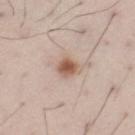A 15 mm crop from a total-body photograph taken for skin-cancer surveillance. The recorded lesion diameter is about 2.5 mm. Imaged with white-light lighting. The patient is a male roughly 55 years of age. An algorithmic analysis of the crop reported border irregularity of about 1.5 on a 0–10 scale, a color-variation rating of about 3.5/10, and radial color variation of about 1. The analysis additionally found an automated nevus-likeness rating near 95 out of 100 and a lesion-detection confidence of about 100/100. On the leg.